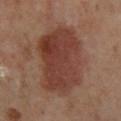{
  "biopsy_status": "not biopsied; imaged during a skin examination",
  "site": "right lower leg",
  "automated_metrics": {
    "area_mm2_approx": 40.0,
    "eccentricity": 0.8,
    "nevus_likeness_0_100": 95,
    "lesion_detection_confidence_0_100": 100
  },
  "patient": {
    "sex": "female",
    "age_approx": 55
  },
  "lesion_size": {
    "long_diameter_mm_approx": 9.0
  },
  "lighting": "cross-polarized",
  "image": {
    "source": "total-body photography crop",
    "field_of_view_mm": 15
  }
}